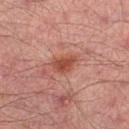* notes: no biopsy performed (imaged during a skin exam)
* diameter: about 3.5 mm
* site: the right lower leg
* tile lighting: cross-polarized
* patient: male, aged 28–32
* automated metrics: a footprint of about 5.5 mm², a shape eccentricity near 0.8, and a shape-asymmetry score of about 0.2 (0 = symmetric); a border-irregularity index near 2/10; a classifier nevus-likeness of about 60/100
* acquisition: ~15 mm tile from a whole-body skin photo